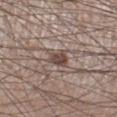| feature | finding |
|---|---|
| notes | catalogued during a skin exam; not biopsied |
| patient | male, roughly 60 years of age |
| lighting | white-light |
| body site | the right lower leg |
| automated lesion analysis | a lesion color around L≈45 a*≈16 b*≈21 in CIELAB and roughly 12 lightness units darker than nearby skin |
| imaging modality | ~15 mm tile from a whole-body skin photo |
| lesion diameter | about 2.5 mm |